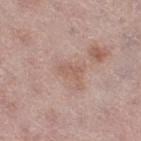This lesion was catalogued during total-body skin photography and was not selected for biopsy.
About 2.5 mm across.
The patient is a female roughly 60 years of age.
This is a white-light tile.
The lesion-visualizer software estimated a mean CIELAB color near L≈57 a*≈19 b*≈26, a lesion–skin lightness drop of about 7, and a lesion-to-skin contrast of about 5 (normalized; higher = more distinct).
A close-up tile cropped from a whole-body skin photograph, about 15 mm across.
The lesion is on the leg.